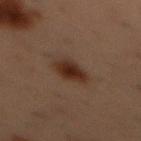Recorded during total-body skin imaging; not selected for excision or biopsy.
The subject is a male about 55 years old.
The lesion is on the chest.
This image is a 15 mm lesion crop taken from a total-body photograph.